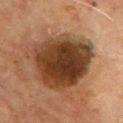Q: Was a biopsy performed?
A: no biopsy performed (imaged during a skin exam)
Q: Where on the body is the lesion?
A: the chest
Q: What are the patient's age and sex?
A: male, aged around 65
Q: What is the lesion's diameter?
A: about 8 mm
Q: What lighting was used for the tile?
A: cross-polarized illumination
Q: What kind of image is this?
A: ~15 mm tile from a whole-body skin photo